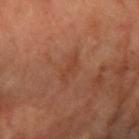tile lighting: cross-polarized illumination
site: the right forearm
patient: female, about 70 years old
image source: 15 mm crop, total-body photography
lesion diameter: ≈3.5 mm